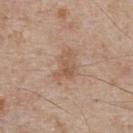notes = catalogued during a skin exam; not biopsied
subject = male, aged approximately 65
lesion diameter = ~4 mm (longest diameter)
image = ~15 mm crop, total-body skin-cancer survey
illumination = white-light
location = the chest